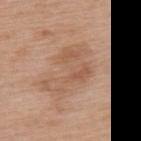Captured during whole-body skin photography for melanoma surveillance; the lesion was not biopsied. Imaged with white-light lighting. From the upper back. The recorded lesion diameter is about 7 mm. A 15 mm close-up tile from a total-body photography series done for melanoma screening. A female subject about 75 years old.